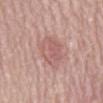<lesion>
<biopsy_status>not biopsied; imaged during a skin examination</biopsy_status>
<patient>
  <sex>female</sex>
  <age_approx>65</age_approx>
</patient>
<lesion_size>
  <long_diameter_mm_approx>5.0</long_diameter_mm_approx>
</lesion_size>
<lighting>white-light</lighting>
<image>
  <source>total-body photography crop</source>
  <field_of_view_mm>15</field_of_view_mm>
</image>
<site>mid back</site>
<automated_metrics>
  <cielab_L>59</cielab_L>
  <cielab_a>22</cielab_a>
  <cielab_b>23</cielab_b>
  <vs_skin_contrast_norm>5.0</vs_skin_contrast_norm>
</automated_metrics>
</lesion>